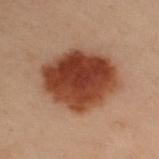Notes:
• workup: no biopsy performed (imaged during a skin exam)